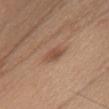Clinical impression: The lesion was photographed on a routine skin check and not biopsied; there is no pathology result. Context: A female subject in their 50s. Imaged with white-light lighting. Cropped from a whole-body photographic skin survey; the tile spans about 15 mm. Longest diameter approximately 3 mm. The lesion is on the chest. The total-body-photography lesion software estimated a lesion area of about 4.5 mm², a shape eccentricity near 0.8, and a symmetry-axis asymmetry near 0.3. And it measured a lesion color around L≈50 a*≈20 b*≈30 in CIELAB, roughly 9 lightness units darker than nearby skin, and a normalized lesion–skin contrast near 6.5. The software also gave a classifier nevus-likeness of about 55/100 and lesion-presence confidence of about 100/100.